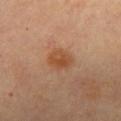biopsy_status: not biopsied; imaged during a skin examination
image:
  source: total-body photography crop
  field_of_view_mm: 15
patient:
  sex: female
  age_approx: 65
site: chest
lighting: cross-polarized